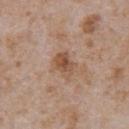{"biopsy_status": "not biopsied; imaged during a skin examination", "automated_metrics": {"cielab_L": 51, "cielab_a": 20, "cielab_b": 30, "vs_skin_darker_L": 10.0, "vs_skin_contrast_norm": 7.5, "nevus_likeness_0_100": 5, "lesion_detection_confidence_0_100": 100}, "site": "chest", "lighting": "white-light", "lesion_size": {"long_diameter_mm_approx": 3.0}, "image": {"source": "total-body photography crop", "field_of_view_mm": 15}, "patient": {"sex": "male", "age_approx": 65}}